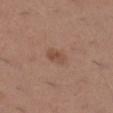Impression: No biopsy was performed on this lesion — it was imaged during a full skin examination and was not determined to be concerning. Acquisition and patient details: Imaged with white-light lighting. The lesion is located on the right lower leg. A 15 mm close-up tile from a total-body photography series done for melanoma screening. Approximately 2.5 mm at its widest. A female subject, aged 28 to 32.